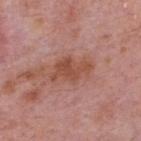No biopsy was performed on this lesion — it was imaged during a full skin examination and was not determined to be concerning.
The recorded lesion diameter is about 5 mm.
A lesion tile, about 15 mm wide, cut from a 3D total-body photograph.
From the upper back.
This is a white-light tile.
The subject is a male about 75 years old.